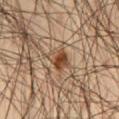| feature | finding |
|---|---|
| lesion size | ~3.5 mm (longest diameter) |
| patient | male, roughly 45 years of age |
| lighting | cross-polarized |
| image source | ~15 mm crop, total-body skin-cancer survey |
| location | the left thigh |